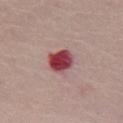workup = catalogued during a skin exam; not biopsied | body site = the chest | illumination = white-light illumination | subject = female, aged 63–67 | image = ~15 mm tile from a whole-body skin photo.